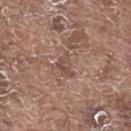Image and clinical context: A close-up tile cropped from a whole-body skin photograph, about 15 mm across. The patient is a male roughly 80 years of age. This is a white-light tile. The lesion is on the upper back. The lesion's longest dimension is about 3 mm.